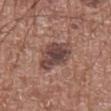Case summary:
– workup — total-body-photography surveillance lesion; no biopsy
– imaging modality — ~15 mm crop, total-body skin-cancer survey
– body site — the chest
– lighting — white-light illumination
– image-analysis metrics — a border-irregularity rating of about 3/10, a within-lesion color-variation index near 5/10, and peripheral color asymmetry of about 1.5; a classifier nevus-likeness of about 30/100 and a detector confidence of about 100 out of 100 that the crop contains a lesion
– patient — male, aged 73 to 77